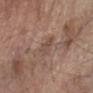Captured during whole-body skin photography for melanoma surveillance; the lesion was not biopsied. On the arm. A 15 mm close-up tile from a total-body photography series done for melanoma screening. A male subject about 60 years old.